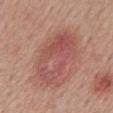follow-up: no biopsy performed (imaged during a skin exam) | imaging modality: 15 mm crop, total-body photography | patient: male, in their mid-50s | lesion diameter: about 6.5 mm | site: the mid back.